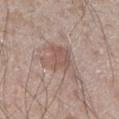| key | value |
|---|---|
| workup | total-body-photography surveillance lesion; no biopsy |
| lesion diameter | about 5 mm |
| subject | male, aged 33–37 |
| image-analysis metrics | a border-irregularity rating of about 6/10, a color-variation rating of about 3/10, and a peripheral color-asymmetry measure near 1 |
| anatomic site | the right lower leg |
| tile lighting | white-light |
| image | total-body-photography crop, ~15 mm field of view |